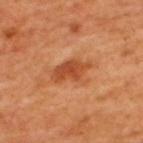The subject is a male aged approximately 50. The tile uses cross-polarized illumination. A region of skin cropped from a whole-body photographic capture, roughly 15 mm wide. Automated tile analysis of the lesion measured a shape eccentricity near 0.8 and a symmetry-axis asymmetry near 0.35. And it measured a border-irregularity rating of about 4.5/10 and peripheral color asymmetry of about 1.5. Longest diameter approximately 4.5 mm. The lesion is on the upper back.